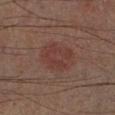biopsy status: imaged on a skin check; not biopsied
tile lighting: cross-polarized illumination
location: the left lower leg
image source: 15 mm crop, total-body photography
patient: male, in their 50s
TBP lesion metrics: a mean CIELAB color near L≈33 a*≈19 b*≈20, about 5 CIELAB-L* units darker than the surrounding skin, and a normalized border contrast of about 5
lesion diameter: about 4.5 mm